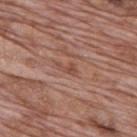Q: Was this lesion biopsied?
A: total-body-photography surveillance lesion; no biopsy
Q: Automated lesion metrics?
A: about 7 CIELAB-L* units darker than the surrounding skin; a border-irregularity index near 5.5/10 and peripheral color asymmetry of about 0; an automated nevus-likeness rating near 0 out of 100 and a lesion-detection confidence of about 100/100
Q: What is the lesion's diameter?
A: ≈2.5 mm
Q: What kind of image is this?
A: total-body-photography crop, ~15 mm field of view
Q: What lighting was used for the tile?
A: white-light illumination
Q: Lesion location?
A: the mid back
Q: Patient demographics?
A: male, aged 68 to 72